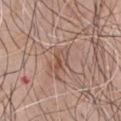Assessment: Captured during whole-body skin photography for melanoma surveillance; the lesion was not biopsied. Background: The lesion's longest dimension is about 4 mm. The lesion is on the chest. Cropped from a whole-body photographic skin survey; the tile spans about 15 mm. A male subject, aged 68–72.